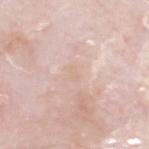The lesion was tiled from a total-body skin photograph and was not biopsied. The patient is a male roughly 75 years of age. Cropped from a total-body skin-imaging series; the visible field is about 15 mm. Captured under white-light illumination. An algorithmic analysis of the crop reported a border-irregularity index near 3.5/10 and radial color variation of about 0. The analysis additionally found a classifier nevus-likeness of about 0/100 and a lesion-detection confidence of about 90/100. The recorded lesion diameter is about 1.5 mm. On the head or neck.